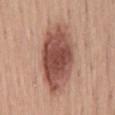<record>
<biopsy_status>not biopsied; imaged during a skin examination</biopsy_status>
<patient>
  <sex>male</sex>
  <age_approx>45</age_approx>
</patient>
<image>
  <source>total-body photography crop</source>
  <field_of_view_mm>15</field_of_view_mm>
</image>
<automated_metrics>
  <vs_skin_contrast_norm>10.5</vs_skin_contrast_norm>
</automated_metrics>
<lighting>white-light</lighting>
<site>lower back</site>
</record>